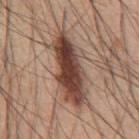Clinical impression: No biopsy was performed on this lesion — it was imaged during a full skin examination and was not determined to be concerning. Clinical summary: The total-body-photography lesion software estimated a footprint of about 21 mm² and a symmetry-axis asymmetry near 0.2. It also reported a mean CIELAB color near L≈43 a*≈20 b*≈25, a lesion–skin lightness drop of about 17, and a lesion-to-skin contrast of about 12.5 (normalized; higher = more distinct). The analysis additionally found border irregularity of about 3.5 on a 0–10 scale, a color-variation rating of about 7.5/10, and peripheral color asymmetry of about 2.5. The software also gave an automated nevus-likeness rating near 95 out of 100 and lesion-presence confidence of about 100/100. A male subject, roughly 60 years of age. Captured under white-light illumination. This image is a 15 mm lesion crop taken from a total-body photograph. Measured at roughly 8.5 mm in maximum diameter. The lesion is located on the abdomen.